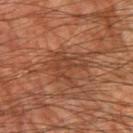Findings:
• biopsy status — catalogued during a skin exam; not biopsied
• lighting — cross-polarized illumination
• subject — male, in their 60s
• size — about 4 mm
• imaging modality — ~15 mm tile from a whole-body skin photo
• location — the right upper arm